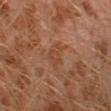Impression: Imaged during a routine full-body skin examination; the lesion was not biopsied and no histopathology is available. Clinical summary: A male subject aged 28–32. A close-up tile cropped from a whole-body skin photograph, about 15 mm across. Longest diameter approximately 3 mm. The lesion is on the leg. Automated image analysis of the tile measured an average lesion color of about L≈43 a*≈22 b*≈31 (CIELAB), about 5 CIELAB-L* units darker than the surrounding skin, and a normalized lesion–skin contrast near 4.5. It also reported a border-irregularity rating of about 5/10 and a color-variation rating of about 1.5/10. It also reported a nevus-likeness score of about 0/100 and a lesion-detection confidence of about 100/100.